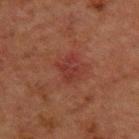Acquisition and patient details:
A lesion tile, about 15 mm wide, cut from a 3D total-body photograph. The lesion is on the back. A male subject roughly 50 years of age. Captured under cross-polarized illumination.